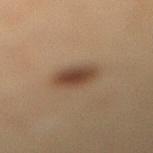  biopsy_status: not biopsied; imaged during a skin examination